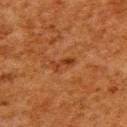workup — total-body-photography surveillance lesion; no biopsy | image — ~15 mm tile from a whole-body skin photo | patient — female, aged approximately 50 | image-analysis metrics — a footprint of about 2.5 mm², an eccentricity of roughly 0.95, and two-axis asymmetry of about 0.45; a nevus-likeness score of about 0/100 and a lesion-detection confidence of about 100/100 | lighting — cross-polarized illumination | location — the upper back.